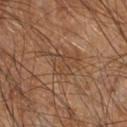Case summary:
– tile lighting — cross-polarized illumination
– image source — 15 mm crop, total-body photography
– lesion diameter — ≈3.5 mm
– patient — male, aged around 60
– anatomic site — the right forearm